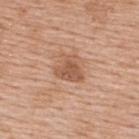Impression:
The lesion was photographed on a routine skin check and not biopsied; there is no pathology result.
Image and clinical context:
The lesion is located on the back. Approximately 3.5 mm at its widest. A female subject, aged approximately 65. Captured under white-light illumination. A 15 mm crop from a total-body photograph taken for skin-cancer surveillance.